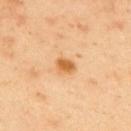A male patient in their mid- to late 40s. The tile uses cross-polarized illumination. A region of skin cropped from a whole-body photographic capture, roughly 15 mm wide. The lesion is on the upper back. About 2.5 mm across.